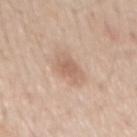This lesion was catalogued during total-body skin photography and was not selected for biopsy. The patient is a male aged 58 to 62. The lesion's longest dimension is about 3.5 mm. The tile uses white-light illumination. On the mid back. Cropped from a total-body skin-imaging series; the visible field is about 15 mm.